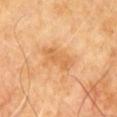Clinical impression:
Captured during whole-body skin photography for melanoma surveillance; the lesion was not biopsied.
Background:
From the upper back. A close-up tile cropped from a whole-body skin photograph, about 15 mm across. A male patient aged around 60.